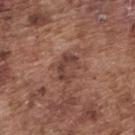Impression:
The lesion was photographed on a routine skin check and not biopsied; there is no pathology result.
Background:
Cropped from a whole-body photographic skin survey; the tile spans about 15 mm. On the upper back. A male patient, approximately 75 years of age.